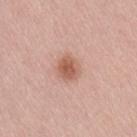Imaged during a routine full-body skin examination; the lesion was not biopsied and no histopathology is available.